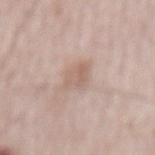{
  "biopsy_status": "not biopsied; imaged during a skin examination",
  "site": "mid back",
  "automated_metrics": {
    "cielab_L": 62,
    "cielab_a": 16,
    "cielab_b": 25,
    "vs_skin_darker_L": 8.0,
    "vs_skin_contrast_norm": 5.5,
    "border_irregularity_0_10": 2.5,
    "color_variation_0_10": 2.5,
    "peripheral_color_asymmetry": 1.0
  },
  "patient": {
    "sex": "male",
    "age_approx": 65
  },
  "image": {
    "source": "total-body photography crop",
    "field_of_view_mm": 15
  },
  "lesion_size": {
    "long_diameter_mm_approx": 3.0
  },
  "lighting": "white-light"
}